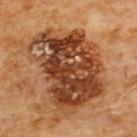biopsy status: catalogued during a skin exam; not biopsied
lighting: cross-polarized
lesion size: ≈9.5 mm
body site: the chest
image-analysis metrics: a lesion area of about 55 mm² and an outline eccentricity of about 0.7 (0 = round, 1 = elongated); a border-irregularity index near 3.5/10, a color-variation rating of about 10/10, and peripheral color asymmetry of about 4
subject: male, in their 60s
image: ~15 mm tile from a whole-body skin photo